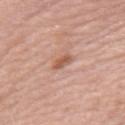Part of a total-body skin-imaging series; this lesion was reviewed on a skin check and was not flagged for biopsy. The lesion is on the right upper arm. A female patient aged 63 to 67. A region of skin cropped from a whole-body photographic capture, roughly 15 mm wide.